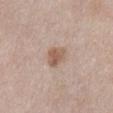Assessment:
The lesion was photographed on a routine skin check and not biopsied; there is no pathology result.